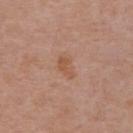Impression:
Captured during whole-body skin photography for melanoma surveillance; the lesion was not biopsied.
Context:
The subject is a male approximately 70 years of age. A 15 mm close-up tile from a total-body photography series done for melanoma screening. On the abdomen.